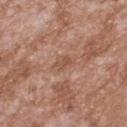<case>
<biopsy_status>not biopsied; imaged during a skin examination</biopsy_status>
<site>upper back</site>
<image>
  <source>total-body photography crop</source>
  <field_of_view_mm>15</field_of_view_mm>
</image>
<lesion_size>
  <long_diameter_mm_approx>3.0</long_diameter_mm_approx>
</lesion_size>
<automated_metrics>
  <cielab_L>52</cielab_L>
  <cielab_a>22</cielab_a>
  <cielab_b>30</cielab_b>
  <vs_skin_darker_L>7.0</vs_skin_darker_L>
  <vs_skin_contrast_norm>5.0</vs_skin_contrast_norm>
  <border_irregularity_0_10>3.0</border_irregularity_0_10>
  <color_variation_0_10>2.5</color_variation_0_10>
  <peripheral_color_asymmetry>1.0</peripheral_color_asymmetry>
  <nevus_likeness_0_100>0</nevus_likeness_0_100>
  <lesion_detection_confidence_0_100>95</lesion_detection_confidence_0_100>
</automated_metrics>
<patient>
  <sex>male</sex>
  <age_approx>45</age_approx>
</patient>
</case>